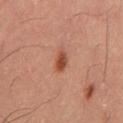Imaged during a routine full-body skin examination; the lesion was not biopsied and no histopathology is available.
About 2.5 mm across.
Located on the abdomen.
A 15 mm crop from a total-body photograph taken for skin-cancer surveillance.
Automated tile analysis of the lesion measured border irregularity of about 1.5 on a 0–10 scale, a within-lesion color-variation index near 1.5/10, and a peripheral color-asymmetry measure near 0.5. The analysis additionally found a nevus-likeness score of about 100/100.
Imaged with cross-polarized lighting.
A male patient in their mid-50s.